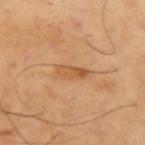Impression:
No biopsy was performed on this lesion — it was imaged during a full skin examination and was not determined to be concerning.